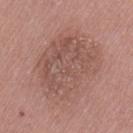The lesion was tiled from a total-body skin photograph and was not biopsied.
Longest diameter approximately 7.5 mm.
A female patient approximately 60 years of age.
A lesion tile, about 15 mm wide, cut from a 3D total-body photograph.
Captured under white-light illumination.
Located on the left thigh.